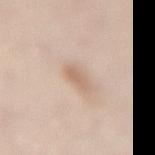No biopsy was performed on this lesion — it was imaged during a full skin examination and was not determined to be concerning.
A female subject, aged approximately 50.
Longest diameter approximately 3 mm.
A 15 mm close-up extracted from a 3D total-body photography capture.
Located on the lower back.